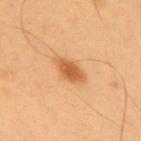Assessment:
The lesion was photographed on a routine skin check and not biopsied; there is no pathology result.